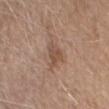Captured during whole-body skin photography for melanoma surveillance; the lesion was not biopsied. Cropped from a whole-body photographic skin survey; the tile spans about 15 mm. On the head or neck. The lesion's longest dimension is about 3.5 mm. The subject is a male in their mid-60s. The tile uses white-light illumination.